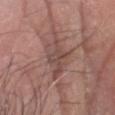  biopsy_status: not biopsied; imaged during a skin examination
  lesion_size:
    long_diameter_mm_approx: 5.0
  image:
    source: total-body photography crop
    field_of_view_mm: 15
  site: head or neck
  automated_metrics:
    area_mm2_approx: 12.0
    eccentricity: 0.6
    shape_asymmetry: 0.25
    cielab_L: 47
    cielab_a: 19
    cielab_b: 22
    vs_skin_contrast_norm: 5.5
    border_irregularity_0_10: 3.5
    color_variation_0_10: 4.0
    peripheral_color_asymmetry: 1.5
    nevus_likeness_0_100: 0
    lesion_detection_confidence_0_100: 60
  patient:
    sex: male
    age_approx: 60